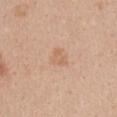workup: imaged on a skin check; not biopsied.